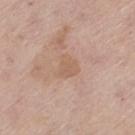| feature | finding |
|---|---|
| follow-up | imaged on a skin check; not biopsied |
| diameter | about 2.5 mm |
| automated metrics | a border-irregularity rating of about 3/10 and peripheral color asymmetry of about 1; an automated nevus-likeness rating near 0 out of 100 |
| patient | female, aged 63 to 67 |
| site | the left thigh |
| acquisition | 15 mm crop, total-body photography |